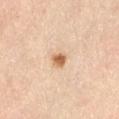follow-up = catalogued during a skin exam; not biopsied.